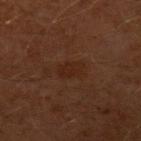Recorded during total-body skin imaging; not selected for excision or biopsy. Automated image analysis of the tile measured a border-irregularity rating of about 2.5/10 and peripheral color asymmetry of about 0.5. The software also gave a nevus-likeness score of about 15/100. Measured at roughly 3 mm in maximum diameter. Located on the arm. A male patient, aged approximately 60. Cropped from a total-body skin-imaging series; the visible field is about 15 mm.